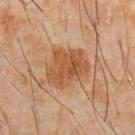Impression:
Captured during whole-body skin photography for melanoma surveillance; the lesion was not biopsied.
Clinical summary:
Imaged with cross-polarized lighting. Cropped from a whole-body photographic skin survey; the tile spans about 15 mm. A male subject, in their 60s. The recorded lesion diameter is about 5 mm. The total-body-photography lesion software estimated a within-lesion color-variation index near 3.5/10. It also reported a classifier nevus-likeness of about 40/100 and a lesion-detection confidence of about 100/100. The lesion is on the abdomen.